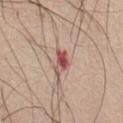notes = total-body-photography surveillance lesion; no biopsy | tile lighting = white-light | automated metrics = an area of roughly 4.5 mm², an eccentricity of roughly 0.8, and two-axis asymmetry of about 0.45; a mean CIELAB color near L≈53 a*≈25 b*≈23 and a normalized lesion–skin contrast near 9.5; a border-irregularity index near 4.5/10, internal color variation of about 5 on a 0–10 scale, and peripheral color asymmetry of about 1.5; a nevus-likeness score of about 0/100 and lesion-presence confidence of about 100/100 | body site = the abdomen | imaging modality = 15 mm crop, total-body photography | lesion size = about 3 mm | patient = female, in their mid-60s.